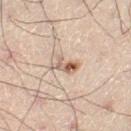Image and clinical context: The patient is a male in their 50s. Imaged with cross-polarized lighting. An algorithmic analysis of the crop reported a mean CIELAB color near L≈53 a*≈15 b*≈26 and roughly 12 lightness units darker than nearby skin. And it measured border irregularity of about 5 on a 0–10 scale, a color-variation rating of about 4/10, and peripheral color asymmetry of about 1.5. A region of skin cropped from a whole-body photographic capture, roughly 15 mm wide. About 3.5 mm across. The lesion is located on the leg.